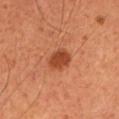Case summary:
- patient · male, roughly 50 years of age
- size · ~3 mm (longest diameter)
- illumination · cross-polarized
- image · ~15 mm tile from a whole-body skin photo
- anatomic site · the left upper arm